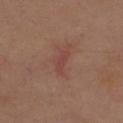<lesion>
<biopsy_status>not biopsied; imaged during a skin examination</biopsy_status>
<image>
  <source>total-body photography crop</source>
  <field_of_view_mm>15</field_of_view_mm>
</image>
<site>upper back</site>
<patient>
  <sex>male</sex>
  <age_approx>70</age_approx>
</patient>
<automated_metrics>
  <shape_asymmetry>0.4</shape_asymmetry>
  <cielab_L>43</cielab_L>
  <cielab_a>24</cielab_a>
  <cielab_b>24</cielab_b>
  <vs_skin_darker_L>6.0</vs_skin_darker_L>
  <vs_skin_contrast_norm>5.0</vs_skin_contrast_norm>
  <color_variation_0_10>0.0</color_variation_0_10>
  <peripheral_color_asymmetry>0.0</peripheral_color_asymmetry>
</automated_metrics>
</lesion>